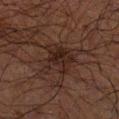follow-up = imaged on a skin check; not biopsied
acquisition = 15 mm crop, total-body photography
subject = male, aged 68 to 72
site = the left forearm